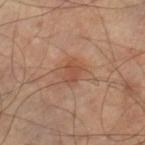biopsy_status: not biopsied; imaged during a skin examination
lighting: cross-polarized
site: right thigh
lesion_size:
  long_diameter_mm_approx: 2.5
image:
  source: total-body photography crop
  field_of_view_mm: 15